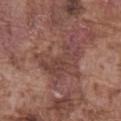Assessment: Recorded during total-body skin imaging; not selected for excision or biopsy. Clinical summary: The patient is a male approximately 75 years of age. From the abdomen. Cropped from a total-body skin-imaging series; the visible field is about 15 mm.